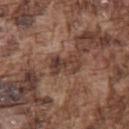biopsy_status: not biopsied; imaged during a skin examination
automated_metrics:
  cielab_L: 38
  cielab_a: 19
  cielab_b: 24
  vs_skin_darker_L: 10.0
  border_irregularity_0_10: 6.0
  color_variation_0_10: 2.5
  peripheral_color_asymmetry: 0.5
  nevus_likeness_0_100: 0
  lesion_detection_confidence_0_100: 70
site: left upper arm
lighting: white-light
image:
  source: total-body photography crop
  field_of_view_mm: 15
patient:
  sex: male
  age_approx: 75